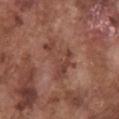notes=catalogued during a skin exam; not biopsied
subject=male, roughly 75 years of age
acquisition=~15 mm crop, total-body skin-cancer survey
site=the chest